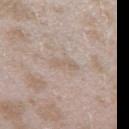Q: Is there a histopathology result?
A: no biopsy performed (imaged during a skin exam)
Q: What is the lesion's diameter?
A: about 3 mm
Q: What is the anatomic site?
A: the left forearm
Q: How was the tile lit?
A: white-light illumination
Q: How was this image acquired?
A: total-body-photography crop, ~15 mm field of view
Q: What are the patient's age and sex?
A: female, aged 23 to 27